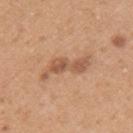{
  "biopsy_status": "not biopsied; imaged during a skin examination",
  "patient": {
    "sex": "female",
    "age_approx": 40
  },
  "lighting": "white-light",
  "site": "left upper arm",
  "lesion_size": {
    "long_diameter_mm_approx": 6.5
  },
  "image": {
    "source": "total-body photography crop",
    "field_of_view_mm": 15
  }
}